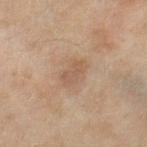Q: Is there a histopathology result?
A: catalogued during a skin exam; not biopsied
Q: What is the imaging modality?
A: ~15 mm tile from a whole-body skin photo
Q: What is the lesion's diameter?
A: about 3.5 mm
Q: What is the anatomic site?
A: the right thigh
Q: What lighting was used for the tile?
A: cross-polarized illumination
Q: Who is the patient?
A: female, aged around 60